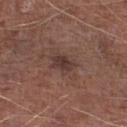Assessment:
Imaged during a routine full-body skin examination; the lesion was not biopsied and no histopathology is available.
Context:
A close-up tile cropped from a whole-body skin photograph, about 15 mm across. From the right lower leg. The subject is a male in their mid- to late 70s.